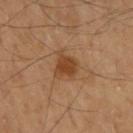notes: total-body-photography surveillance lesion; no biopsy
patient: male, approximately 55 years of age
lighting: cross-polarized
body site: the upper back
acquisition: total-body-photography crop, ~15 mm field of view